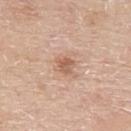workup — imaged on a skin check; not biopsied | size — ≈2.5 mm | image source — total-body-photography crop, ~15 mm field of view | location — the arm | subject — male, roughly 80 years of age | lighting — white-light illumination.